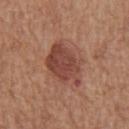– biopsy status · imaged on a skin check; not biopsied
– acquisition · total-body-photography crop, ~15 mm field of view
– patient · male, in their mid- to late 60s
– diameter · ≈5.5 mm
– TBP lesion metrics · an area of roughly 16 mm², an outline eccentricity of about 0.7 (0 = round, 1 = elongated), and two-axis asymmetry of about 0.2; a lesion color around L≈45 a*≈24 b*≈27 in CIELAB and a lesion-to-skin contrast of about 8.5 (normalized; higher = more distinct); a border-irregularity index near 3/10 and internal color variation of about 3.5 on a 0–10 scale; a nevus-likeness score of about 70/100 and a detector confidence of about 100 out of 100 that the crop contains a lesion
– location · the chest